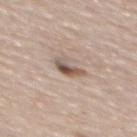  biopsy_status: not biopsied; imaged during a skin examination
  lesion_size:
    long_diameter_mm_approx: 3.0
  lighting: white-light
  image:
    source: total-body photography crop
    field_of_view_mm: 15
  site: upper back
  patient:
    sex: female
    age_approx: 65